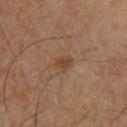| field | value |
|---|---|
| notes | imaged on a skin check; not biopsied |
| subject | male, aged around 55 |
| lighting | cross-polarized |
| body site | the front of the torso |
| image source | ~15 mm crop, total-body skin-cancer survey |